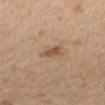Q: Was a biopsy performed?
A: imaged on a skin check; not biopsied
Q: What is the lesion's diameter?
A: about 3.5 mm
Q: What is the imaging modality?
A: total-body-photography crop, ~15 mm field of view
Q: What is the anatomic site?
A: the mid back
Q: What are the patient's age and sex?
A: female, approximately 70 years of age